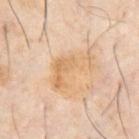<lesion>
  <biopsy_status>not biopsied; imaged during a skin examination</biopsy_status>
  <image>
    <source>total-body photography crop</source>
    <field_of_view_mm>15</field_of_view_mm>
  </image>
  <lesion_size>
    <long_diameter_mm_approx>6.5</long_diameter_mm_approx>
  </lesion_size>
  <lighting>cross-polarized</lighting>
  <patient>
    <sex>male</sex>
    <age_approx>60</age_approx>
  </patient>
  <site>abdomen</site>
  <automated_metrics>
    <border_irregularity_0_10>9.5</border_irregularity_0_10>
  </automated_metrics>
</lesion>